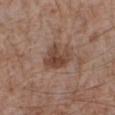Notes:
• biopsy status: catalogued during a skin exam; not biopsied
• image-analysis metrics: internal color variation of about 4.5 on a 0–10 scale; a classifier nevus-likeness of about 65/100
• lesion size: ~4 mm (longest diameter)
• subject: male, aged 73 to 77
• image: ~15 mm crop, total-body skin-cancer survey
• lighting: white-light
• site: the right forearm